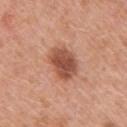| key | value |
|---|---|
| workup | catalogued during a skin exam; not biopsied |
| patient | female, aged 38 to 42 |
| site | the right upper arm |
| tile lighting | white-light |
| size | about 4 mm |
| image-analysis metrics | a footprint of about 11 mm², an outline eccentricity of about 0.6 (0 = round, 1 = elongated), and a symmetry-axis asymmetry near 0.15; border irregularity of about 1.5 on a 0–10 scale, a color-variation rating of about 4/10, and peripheral color asymmetry of about 1; a classifier nevus-likeness of about 85/100 and lesion-presence confidence of about 100/100 |
| image source | total-body-photography crop, ~15 mm field of view |